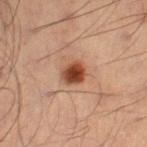Case summary:
* workup · catalogued during a skin exam; not biopsied
* body site · the left leg
* subject · male, approximately 50 years of age
* image source · ~15 mm crop, total-body skin-cancer survey